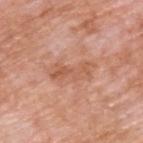This lesion was catalogued during total-body skin photography and was not selected for biopsy.